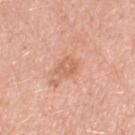workup = catalogued during a skin exam; not biopsied | size = ≈2.5 mm | image source = ~15 mm tile from a whole-body skin photo | subject = female, in their 70s | TBP lesion metrics = a footprint of about 4.5 mm² and a symmetry-axis asymmetry near 0.25; a classifier nevus-likeness of about 0/100 and a lesion-detection confidence of about 100/100 | lighting = white-light | site = the left thigh.